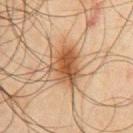workup — no biopsy performed (imaged during a skin exam)
lighting — cross-polarized
lesion size — ~5 mm (longest diameter)
subject — male, aged 43–47
image — ~15 mm tile from a whole-body skin photo
site — the chest